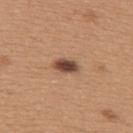| key | value |
|---|---|
| workup | no biopsy performed (imaged during a skin exam) |
| body site | the upper back |
| illumination | white-light illumination |
| patient | female, in their 30s |
| size | about 3 mm |
| image | ~15 mm crop, total-body skin-cancer survey |